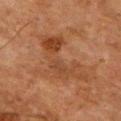• automated lesion analysis · a normalized border contrast of about 6; radial color variation of about 1.5; a classifier nevus-likeness of about 0/100 and lesion-presence confidence of about 100/100
• tile lighting · cross-polarized
• subject · male, aged 63 to 67
• lesion diameter · ≈8.5 mm
• image source · total-body-photography crop, ~15 mm field of view
• body site · the front of the torso